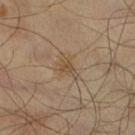Part of a total-body skin-imaging series; this lesion was reviewed on a skin check and was not flagged for biopsy.
On the right lower leg.
A male subject approximately 65 years of age.
A region of skin cropped from a whole-body photographic capture, roughly 15 mm wide.